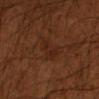Assessment:
No biopsy was performed on this lesion — it was imaged during a full skin examination and was not determined to be concerning.
Background:
This is a cross-polarized tile. A region of skin cropped from a whole-body photographic capture, roughly 15 mm wide. The patient is a male aged around 55. The lesion is on the left forearm. Longest diameter approximately 3.5 mm.